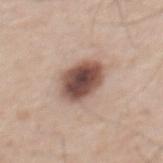Part of a total-body skin-imaging series; this lesion was reviewed on a skin check and was not flagged for biopsy.
On the mid back.
The lesion's longest dimension is about 4.5 mm.
Automated tile analysis of the lesion measured an average lesion color of about L≈50 a*≈19 b*≈24 (CIELAB). And it measured a border-irregularity rating of about 1.5/10 and radial color variation of about 2. The software also gave a lesion-detection confidence of about 100/100.
The patient is a male aged around 65.
Cropped from a total-body skin-imaging series; the visible field is about 15 mm.
The tile uses white-light illumination.